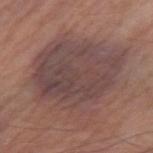Assessment:
This lesion was catalogued during total-body skin photography and was not selected for biopsy.
Acquisition and patient details:
A roughly 15 mm field-of-view crop from a total-body skin photograph. A male subject, in their mid- to late 80s or older. On the right forearm.